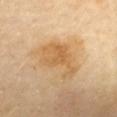Q: Is there a histopathology result?
A: catalogued during a skin exam; not biopsied
Q: Patient demographics?
A: female, in their 60s
Q: What kind of image is this?
A: ~15 mm crop, total-body skin-cancer survey
Q: Illumination type?
A: cross-polarized
Q: Where on the body is the lesion?
A: the mid back
Q: What is the lesion's diameter?
A: ≈5.5 mm
Q: Automated lesion metrics?
A: a lesion color around L≈63 a*≈19 b*≈42 in CIELAB and a normalized border contrast of about 6